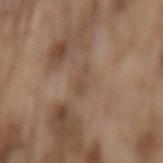| key | value |
|---|---|
| biopsy status | no biopsy performed (imaged during a skin exam) |
| site | the mid back |
| patient | male, roughly 75 years of age |
| image | total-body-photography crop, ~15 mm field of view |
| lesion size | ≈2.5 mm |
| illumination | white-light |